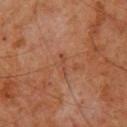Part of a total-body skin-imaging series; this lesion was reviewed on a skin check and was not flagged for biopsy.
Measured at roughly 2.5 mm in maximum diameter.
A male subject, approximately 60 years of age.
Automated tile analysis of the lesion measured an area of roughly 1.5 mm², an outline eccentricity of about 0.95 (0 = round, 1 = elongated), and two-axis asymmetry of about 0.5. The software also gave a border-irregularity index near 5.5/10, internal color variation of about 0 on a 0–10 scale, and radial color variation of about 0. It also reported an automated nevus-likeness rating near 0 out of 100 and lesion-presence confidence of about 100/100.
The lesion is located on the upper back.
A 15 mm close-up tile from a total-body photography series done for melanoma screening.
Captured under cross-polarized illumination.